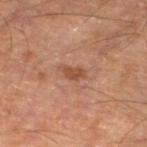Recorded during total-body skin imaging; not selected for excision or biopsy.
The lesion is located on the left lower leg.
This image is a 15 mm lesion crop taken from a total-body photograph.
Captured under cross-polarized illumination.
A male patient aged 53–57.